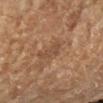Findings:
- follow-up — total-body-photography surveillance lesion; no biopsy
- site — the right forearm
- subject — female, aged 68–72
- illumination — cross-polarized
- image source — 15 mm crop, total-body photography
- diameter — about 3 mm
- automated metrics — an area of roughly 4.5 mm², a shape eccentricity near 0.85, and a shape-asymmetry score of about 0.5 (0 = symmetric); a mean CIELAB color near L≈45 a*≈19 b*≈30, about 6 CIELAB-L* units darker than the surrounding skin, and a normalized border contrast of about 5; border irregularity of about 6 on a 0–10 scale, a within-lesion color-variation index near 1/10, and peripheral color asymmetry of about 0; lesion-presence confidence of about 90/100